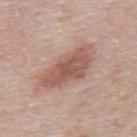Impression:
Recorded during total-body skin imaging; not selected for excision or biopsy.
Clinical summary:
A region of skin cropped from a whole-body photographic capture, roughly 15 mm wide. The patient is a male roughly 55 years of age. Measured at roughly 7 mm in maximum diameter. Automated tile analysis of the lesion measured an area of roughly 18 mm², an outline eccentricity of about 0.9 (0 = round, 1 = elongated), and a shape-asymmetry score of about 0.2 (0 = symmetric). The software also gave a border-irregularity rating of about 3/10 and a peripheral color-asymmetry measure near 1. The lesion is on the back. The tile uses white-light illumination.